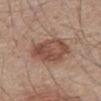| feature | finding |
|---|---|
| notes | imaged on a skin check; not biopsied |
| diameter | ~5 mm (longest diameter) |
| illumination | white-light illumination |
| patient | male, aged approximately 80 |
| image source | ~15 mm tile from a whole-body skin photo |
| automated metrics | a lesion color around L≈49 a*≈20 b*≈26 in CIELAB and a lesion-to-skin contrast of about 8 (normalized; higher = more distinct); a border-irregularity rating of about 2/10, a within-lesion color-variation index near 5/10, and a peripheral color-asymmetry measure near 1.5 |
| body site | the abdomen |